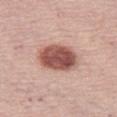| key | value |
|---|---|
| notes | no biopsy performed (imaged during a skin exam) |
| anatomic site | the leg |
| lesion size | about 5 mm |
| lighting | white-light illumination |
| image source | total-body-photography crop, ~15 mm field of view |
| image-analysis metrics | a mean CIELAB color near L≈52 a*≈24 b*≈25, about 18 CIELAB-L* units darker than the surrounding skin, and a normalized lesion–skin contrast near 11.5; an automated nevus-likeness rating near 100 out of 100 and a detector confidence of about 100 out of 100 that the crop contains a lesion |
| subject | female, approximately 65 years of age |